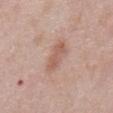Clinical impression:
Part of a total-body skin-imaging series; this lesion was reviewed on a skin check and was not flagged for biopsy.
Image and clinical context:
Measured at roughly 4 mm in maximum diameter. From the abdomen. A close-up tile cropped from a whole-body skin photograph, about 15 mm across. The subject is a male aged around 55.